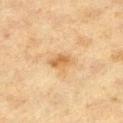lighting = cross-polarized
lesion size = ~2.5 mm (longest diameter)
acquisition = ~15 mm tile from a whole-body skin photo
site = the left thigh
subject = female, approximately 40 years of age